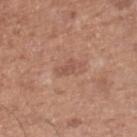{
  "biopsy_status": "not biopsied; imaged during a skin examination",
  "patient": {
    "sex": "male",
    "age_approx": 75
  },
  "lesion_size": {
    "long_diameter_mm_approx": 2.5
  },
  "image": {
    "source": "total-body photography crop",
    "field_of_view_mm": 15
  },
  "lighting": "white-light",
  "site": "right lower leg"
}